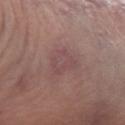Q: Was a biopsy performed?
A: imaged on a skin check; not biopsied
Q: How was the tile lit?
A: white-light illumination
Q: What is the anatomic site?
A: the left forearm
Q: What kind of image is this?
A: 15 mm crop, total-body photography
Q: Patient demographics?
A: male, aged around 65
Q: How large is the lesion?
A: ~4 mm (longest diameter)